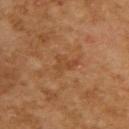Clinical impression: No biopsy was performed on this lesion — it was imaged during a full skin examination and was not determined to be concerning. Context: The lesion is located on the upper back. The recorded lesion diameter is about 3 mm. A close-up tile cropped from a whole-body skin photograph, about 15 mm across. A female subject approximately 60 years of age.